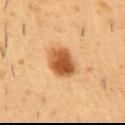notes: total-body-photography surveillance lesion; no biopsy | illumination: cross-polarized | image: total-body-photography crop, ~15 mm field of view | TBP lesion metrics: a border-irregularity index near 1.5/10 and a peripheral color-asymmetry measure near 2; a nevus-likeness score of about 100/100 and a lesion-detection confidence of about 100/100 | patient: male, aged 48 to 52 | location: the abdomen.